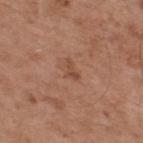Case summary:
* notes — total-body-photography surveillance lesion; no biopsy
* location — the upper back
* lighting — white-light illumination
* patient — male, in their mid- to late 50s
* acquisition — ~15 mm crop, total-body skin-cancer survey
* image-analysis metrics — an automated nevus-likeness rating near 0 out of 100 and lesion-presence confidence of about 100/100